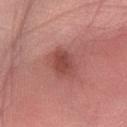This lesion was catalogued during total-body skin photography and was not selected for biopsy.
The subject is a female approximately 40 years of age.
Captured under white-light illumination.
The lesion's longest dimension is about 4 mm.
This image is a 15 mm lesion crop taken from a total-body photograph.
Located on the left forearm.